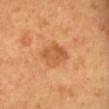The lesion was tiled from a total-body skin photograph and was not biopsied.